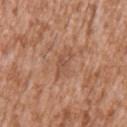Part of a total-body skin-imaging series; this lesion was reviewed on a skin check and was not flagged for biopsy. On the left upper arm. This is a white-light tile. About 3.5 mm across. A lesion tile, about 15 mm wide, cut from a 3D total-body photograph. The patient is a male approximately 45 years of age. An algorithmic analysis of the crop reported a lesion-to-skin contrast of about 5 (normalized; higher = more distinct).